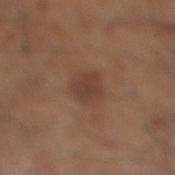An algorithmic analysis of the crop reported a lesion color around L≈40 a*≈19 b*≈25 in CIELAB, about 7 CIELAB-L* units darker than the surrounding skin, and a lesion-to-skin contrast of about 6.5 (normalized; higher = more distinct). The analysis additionally found an automated nevus-likeness rating near 15 out of 100 and a lesion-detection confidence of about 100/100.
Measured at roughly 3.5 mm in maximum diameter.
Imaged with white-light lighting.
From the right lower leg.
A male subject aged around 60.
This image is a 15 mm lesion crop taken from a total-body photograph.